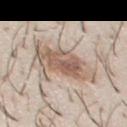biopsy_status: not biopsied; imaged during a skin examination
site: chest
lighting: white-light
patient:
  sex: male
  age_approx: 30
image:
  source: total-body photography crop
  field_of_view_mm: 15